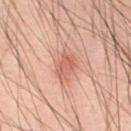Notes:
• follow-up · total-body-photography surveillance lesion; no biopsy
• anatomic site · the abdomen
• acquisition · total-body-photography crop, ~15 mm field of view
• patient · male, roughly 40 years of age